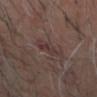This lesion was catalogued during total-body skin photography and was not selected for biopsy. An algorithmic analysis of the crop reported an area of roughly 5 mm², an outline eccentricity of about 0.9 (0 = round, 1 = elongated), and two-axis asymmetry of about 0.3. It also reported border irregularity of about 4.5 on a 0–10 scale and a peripheral color-asymmetry measure near 1.5. On the head or neck. This is a cross-polarized tile. The lesion's longest dimension is about 4 mm. The subject is a male aged approximately 65. A lesion tile, about 15 mm wide, cut from a 3D total-body photograph.